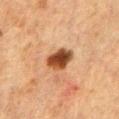Recorded during total-body skin imaging; not selected for excision or biopsy.
The lesion is located on the front of the torso.
Cropped from a total-body skin-imaging series; the visible field is about 15 mm.
Automated tile analysis of the lesion measured a footprint of about 7 mm², an eccentricity of roughly 0.75, and a shape-asymmetry score of about 0.2 (0 = symmetric). And it measured an automated nevus-likeness rating near 100 out of 100 and a detector confidence of about 100 out of 100 that the crop contains a lesion.
Measured at roughly 3.5 mm in maximum diameter.
The tile uses cross-polarized illumination.
A male patient, in their 50s.